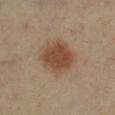Part of a total-body skin-imaging series; this lesion was reviewed on a skin check and was not flagged for biopsy.